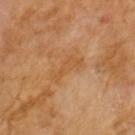Clinical impression: No biopsy was performed on this lesion — it was imaged during a full skin examination and was not determined to be concerning. Background: The total-body-photography lesion software estimated a lesion area of about 5.5 mm², a shape eccentricity near 0.95, and a symmetry-axis asymmetry near 0.45. The software also gave a lesion color around L≈52 a*≈21 b*≈40 in CIELAB, about 6 CIELAB-L* units darker than the surrounding skin, and a normalized lesion–skin contrast near 5.5. The analysis additionally found a nevus-likeness score of about 0/100 and a lesion-detection confidence of about 100/100. This is a cross-polarized tile. The lesion's longest dimension is about 4 mm. The patient is a male approximately 65 years of age. A region of skin cropped from a whole-body photographic capture, roughly 15 mm wide.